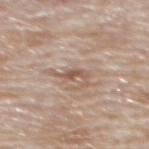The lesion was tiled from a total-body skin photograph and was not biopsied. Located on the back. The lesion's longest dimension is about 2.5 mm. This is a white-light tile. Automated image analysis of the tile measured a lesion area of about 2.5 mm², a shape eccentricity near 0.9, and a symmetry-axis asymmetry near 0.45. The software also gave a lesion color around L≈54 a*≈18 b*≈27 in CIELAB and roughly 10 lightness units darker than nearby skin. A 15 mm close-up extracted from a 3D total-body photography capture. A male subject aged 78–82.